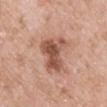Q: Is there a histopathology result?
A: total-body-photography surveillance lesion; no biopsy
Q: What kind of image is this?
A: 15 mm crop, total-body photography
Q: Who is the patient?
A: male, aged 48 to 52
Q: How large is the lesion?
A: ~5 mm (longest diameter)
Q: Lesion location?
A: the chest
Q: What lighting was used for the tile?
A: white-light illumination